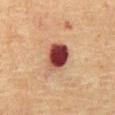Clinical impression:
No biopsy was performed on this lesion — it was imaged during a full skin examination and was not determined to be concerning.
Context:
A 15 mm crop from a total-body photograph taken for skin-cancer surveillance. About 4 mm across. Automated image analysis of the tile measured a border-irregularity rating of about 1.5/10, a within-lesion color-variation index near 9.5/10, and radial color variation of about 3. The analysis additionally found an automated nevus-likeness rating near 0 out of 100. The patient is a male aged 63–67. On the abdomen. Imaged with cross-polarized lighting.